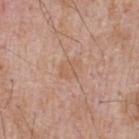Assessment: No biopsy was performed on this lesion — it was imaged during a full skin examination and was not determined to be concerning. Context: The lesion is on the upper back. A close-up tile cropped from a whole-body skin photograph, about 15 mm across. The subject is a male in their mid- to late 60s.